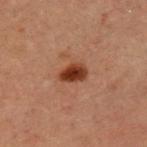biopsy status — catalogued during a skin exam; not biopsied | image source — ~15 mm tile from a whole-body skin photo | patient — male, about 60 years old | anatomic site — the chest | lesion diameter — ~3 mm (longest diameter) | automated metrics — a symmetry-axis asymmetry near 0.25 | illumination — cross-polarized illumination.